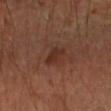The lesion was tiled from a total-body skin photograph and was not biopsied.
A region of skin cropped from a whole-body photographic capture, roughly 15 mm wide.
The lesion is on the left forearm.
A male patient aged approximately 45.
The lesion's longest dimension is about 3 mm.
Imaged with cross-polarized lighting.